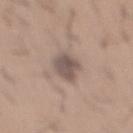Assessment:
This lesion was catalogued during total-body skin photography and was not selected for biopsy.
Clinical summary:
An algorithmic analysis of the crop reported an average lesion color of about L≈53 a*≈13 b*≈20 (CIELAB), roughly 11 lightness units darker than nearby skin, and a normalized border contrast of about 8.5. The analysis additionally found border irregularity of about 2 on a 0–10 scale and radial color variation of about 1. The software also gave a classifier nevus-likeness of about 10/100. A 15 mm close-up tile from a total-body photography series done for melanoma screening. This is a white-light tile. The lesion is located on the mid back. A male patient aged 63 to 67. Longest diameter approximately 4.5 mm.